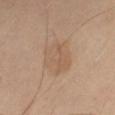About 4.5 mm across. The subject is a female roughly 60 years of age. On the right thigh. Cropped from a total-body skin-imaging series; the visible field is about 15 mm. The tile uses cross-polarized illumination.